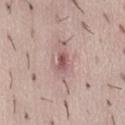Located on the abdomen. Imaged with white-light lighting. The patient is a female in their 40s. A close-up tile cropped from a whole-body skin photograph, about 15 mm across. The lesion's longest dimension is about 2.5 mm.